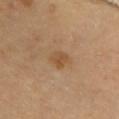Clinical impression:
Imaged during a routine full-body skin examination; the lesion was not biopsied and no histopathology is available.
Clinical summary:
A roughly 15 mm field-of-view crop from a total-body skin photograph. Located on the chest. The patient is a female about 60 years old. The tile uses cross-polarized illumination. The lesion-visualizer software estimated a footprint of about 4.5 mm², an eccentricity of roughly 0.6, and a symmetry-axis asymmetry near 0.25. The analysis additionally found a lesion color around L≈52 a*≈18 b*≈36 in CIELAB and roughly 7 lightness units darker than nearby skin. The analysis additionally found border irregularity of about 2.5 on a 0–10 scale and a color-variation rating of about 2/10. The software also gave an automated nevus-likeness rating near 25 out of 100 and a lesion-detection confidence of about 100/100.